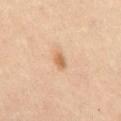Findings:
• follow-up — imaged on a skin check; not biopsied
• diameter — about 2.5 mm
• acquisition — total-body-photography crop, ~15 mm field of view
• automated metrics — a lesion area of about 3 mm² and an eccentricity of roughly 0.75; a lesion color around L≈62 a*≈20 b*≈36 in CIELAB, roughly 10 lightness units darker than nearby skin, and a normalized lesion–skin contrast near 7; border irregularity of about 2.5 on a 0–10 scale, a color-variation rating of about 1/10, and peripheral color asymmetry of about 0.5; a classifier nevus-likeness of about 80/100 and a lesion-detection confidence of about 100/100
• location — the front of the torso
• illumination — cross-polarized
• subject — male, aged 58 to 62